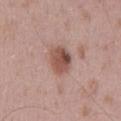Imaged during a routine full-body skin examination; the lesion was not biopsied and no histopathology is available. Measured at roughly 3.5 mm in maximum diameter. A 15 mm close-up tile from a total-body photography series done for melanoma screening. The lesion is on the mid back. The tile uses white-light illumination. The patient is a male aged 48–52.